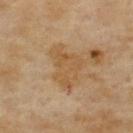Q: What is the imaging modality?
A: total-body-photography crop, ~15 mm field of view
Q: What did automated image analysis measure?
A: a lesion area of about 11 mm² and a shape-asymmetry score of about 0.5 (0 = symmetric); a border-irregularity rating of about 6.5/10, internal color variation of about 2 on a 0–10 scale, and radial color variation of about 0.5; a lesion-detection confidence of about 100/100
Q: What lighting was used for the tile?
A: cross-polarized illumination
Q: What is the lesion's diameter?
A: about 5.5 mm
Q: Where on the body is the lesion?
A: the upper back
Q: Patient demographics?
A: female, aged approximately 60